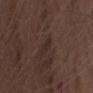Q: Was a biopsy performed?
A: no biopsy performed (imaged during a skin exam)
Q: How large is the lesion?
A: ~2 mm (longest diameter)
Q: Lesion location?
A: the abdomen
Q: What are the patient's age and sex?
A: male, in their 70s
Q: How was this image acquired?
A: total-body-photography crop, ~15 mm field of view
Q: Illumination type?
A: white-light
Q: Automated lesion metrics?
A: an average lesion color of about L≈26 a*≈15 b*≈19 (CIELAB), about 4 CIELAB-L* units darker than the surrounding skin, and a lesion-to-skin contrast of about 5 (normalized; higher = more distinct); border irregularity of about 2.5 on a 0–10 scale